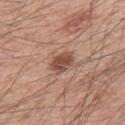notes=no biopsy performed (imaged during a skin exam).